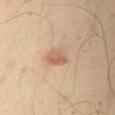Impression: Imaged during a routine full-body skin examination; the lesion was not biopsied and no histopathology is available. Background: The lesion is on the left upper arm. The lesion-visualizer software estimated a lesion area of about 5 mm², an eccentricity of roughly 0.8, and a symmetry-axis asymmetry near 0.25. And it measured a mean CIELAB color near L≈60 a*≈19 b*≈31 and a normalized lesion–skin contrast near 6.5. And it measured a border-irregularity index near 2.5/10 and a within-lesion color-variation index near 4.5/10. A male subject, approximately 50 years of age. Longest diameter approximately 3.5 mm. This is a cross-polarized tile. This image is a 15 mm lesion crop taken from a total-body photograph.